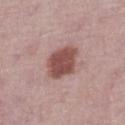Clinical impression: Recorded during total-body skin imaging; not selected for excision or biopsy. Background: Located on the leg. Imaged with white-light lighting. A female patient, about 65 years old. The total-body-photography lesion software estimated a normalized border contrast of about 10. It also reported border irregularity of about 1.5 on a 0–10 scale. The analysis additionally found a classifier nevus-likeness of about 95/100. About 4.5 mm across. Cropped from a whole-body photographic skin survey; the tile spans about 15 mm.